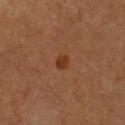  biopsy_status: not biopsied; imaged during a skin examination
  lesion_size:
    long_diameter_mm_approx: 2.0
  image:
    source: total-body photography crop
    field_of_view_mm: 15
  patient:
    sex: male
    age_approx: 60
  site: chest
  lighting: cross-polarized